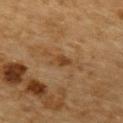* workup: imaged on a skin check; not biopsied
* patient: male, aged 83 or older
* acquisition: total-body-photography crop, ~15 mm field of view
* anatomic site: the mid back
* illumination: cross-polarized
* diameter: ≈3 mm